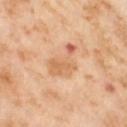  biopsy_status: not biopsied; imaged during a skin examination
  automated_metrics:
    nevus_likeness_0_100: 0
    lesion_detection_confidence_0_100: 100
  site: left thigh
  image:
    source: total-body photography crop
    field_of_view_mm: 15
  patient:
    sex: female
    age_approx: 55
  lighting: cross-polarized
  lesion_size:
    long_diameter_mm_approx: 5.0